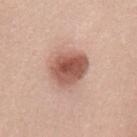anatomic site: the mid back | lighting: white-light | imaging modality: ~15 mm tile from a whole-body skin photo | size: about 5 mm | automated lesion analysis: a lesion area of about 16 mm², a shape eccentricity near 0.65, and a shape-asymmetry score of about 0.2 (0 = symmetric); a lesion color around L≈56 a*≈23 b*≈27 in CIELAB, about 15 CIELAB-L* units darker than the surrounding skin, and a normalized lesion–skin contrast near 9.5; a border-irregularity index near 2/10, a within-lesion color-variation index near 6.5/10, and a peripheral color-asymmetry measure near 2; an automated nevus-likeness rating near 95 out of 100 | subject: female, in their 40s.